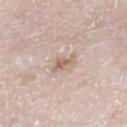biopsy status: catalogued during a skin exam; not biopsied | subject: male, in their 80s | image: 15 mm crop, total-body photography | location: the right lower leg | illumination: white-light illumination | lesion size: ~3 mm (longest diameter).